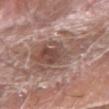Impression:
No biopsy was performed on this lesion — it was imaged during a full skin examination and was not determined to be concerning.
Context:
A region of skin cropped from a whole-body photographic capture, roughly 15 mm wide. The subject is a male roughly 75 years of age. Measured at roughly 8 mm in maximum diameter. An algorithmic analysis of the crop reported a lesion area of about 23 mm², a shape eccentricity near 0.85, and a shape-asymmetry score of about 0.45 (0 = symmetric). The analysis additionally found a lesion color around L≈50 a*≈18 b*≈23 in CIELAB, about 11 CIELAB-L* units darker than the surrounding skin, and a normalized lesion–skin contrast near 7.5. It also reported border irregularity of about 8 on a 0–10 scale and a color-variation rating of about 6/10. The lesion is located on the right forearm.